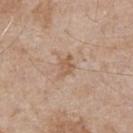follow-up: imaged on a skin check; not biopsied
patient: male, aged around 65
image: ~15 mm tile from a whole-body skin photo
TBP lesion metrics: a footprint of about 3.5 mm², an eccentricity of roughly 0.7, and two-axis asymmetry of about 0.3; a lesion-to-skin contrast of about 6 (normalized; higher = more distinct); a nevus-likeness score of about 0/100 and a lesion-detection confidence of about 100/100
anatomic site: the chest
illumination: white-light